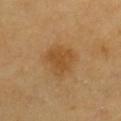biopsy_status: not biopsied; imaged during a skin examination
automated_metrics:
  border_irregularity_0_10: 2.5
  peripheral_color_asymmetry: 0.5
  nevus_likeness_0_100: 85
  lesion_detection_confidence_0_100: 100
lighting: cross-polarized
site: chest
patient:
  sex: male
  age_approx: 60
image:
  source: total-body photography crop
  field_of_view_mm: 15
lesion_size:
  long_diameter_mm_approx: 3.5